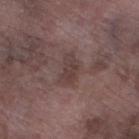workup: imaged on a skin check; not biopsied | site: the leg | acquisition: 15 mm crop, total-body photography | patient: male, in their mid- to late 70s.